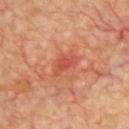Imaged during a routine full-body skin examination; the lesion was not biopsied and no histopathology is available. A region of skin cropped from a whole-body photographic capture, roughly 15 mm wide. The lesion is on the chest. The subject is a male aged 58–62.